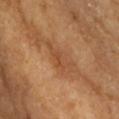{"biopsy_status": "not biopsied; imaged during a skin examination"}